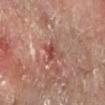  image:
    source: total-body photography crop
    field_of_view_mm: 15
  lighting: cross-polarized
  site: right forearm
  lesion_size:
    long_diameter_mm_approx: 3.0
  patient:
    sex: male
    age_approx: 60